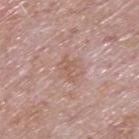{
  "biopsy_status": "not biopsied; imaged during a skin examination",
  "site": "upper back",
  "image": {
    "source": "total-body photography crop",
    "field_of_view_mm": 15
  },
  "patient": {
    "sex": "male",
    "age_approx": 65
  }
}